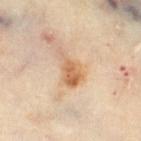Clinical impression: Recorded during total-body skin imaging; not selected for excision or biopsy. Context: About 4.5 mm across. A 15 mm crop from a total-body photograph taken for skin-cancer surveillance. The lesion is on the right thigh. A female patient in their 60s.